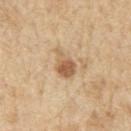Clinical impression: Part of a total-body skin-imaging series; this lesion was reviewed on a skin check and was not flagged for biopsy. Clinical summary: The tile uses white-light illumination. The patient is a male aged approximately 70. Located on the right upper arm. An algorithmic analysis of the crop reported a footprint of about 5.5 mm², an outline eccentricity of about 0.75 (0 = round, 1 = elongated), and a symmetry-axis asymmetry near 0.35. A 15 mm crop from a total-body photograph taken for skin-cancer surveillance.